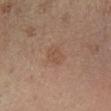| field | value |
|---|---|
| notes | imaged on a skin check; not biopsied |
| site | the left lower leg |
| subject | male, aged 58–62 |
| lesion diameter | ~3 mm (longest diameter) |
| tile lighting | cross-polarized |
| image | 15 mm crop, total-body photography |